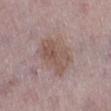The lesion was photographed on a routine skin check and not biopsied; there is no pathology result. The lesion is located on the left lower leg. This image is a 15 mm lesion crop taken from a total-body photograph. A female subject, aged around 50. Imaged with white-light lighting. Measured at roughly 5 mm in maximum diameter.